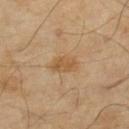Assessment: This lesion was catalogued during total-body skin photography and was not selected for biopsy. Acquisition and patient details: A 15 mm crop from a total-body photograph taken for skin-cancer surveillance. The patient is a male aged 68–72. Approximately 3 mm at its widest. Captured under cross-polarized illumination. The lesion is located on the left lower leg.